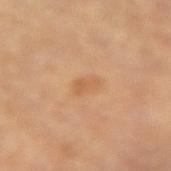Located on the right forearm.
Cropped from a whole-body photographic skin survey; the tile spans about 15 mm.
The lesion-visualizer software estimated an automated nevus-likeness rating near 5 out of 100 and lesion-presence confidence of about 100/100.
The recorded lesion diameter is about 2.5 mm.
A male subject, roughly 85 years of age or older.